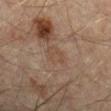| feature | finding |
|---|---|
| follow-up | no biopsy performed (imaged during a skin exam) |
| lesion diameter | about 2.5 mm |
| body site | the arm |
| lighting | cross-polarized |
| image source | ~15 mm crop, total-body skin-cancer survey |
| patient | male, aged 43–47 |